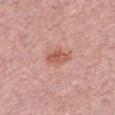The lesion is on the chest. A region of skin cropped from a whole-body photographic capture, roughly 15 mm wide. The subject is a female about 30 years old.